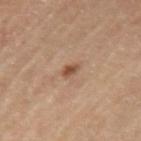{
  "lighting": "cross-polarized",
  "patient": {
    "sex": "female",
    "age_approx": 65
  },
  "lesion_size": {
    "long_diameter_mm_approx": 2.0
  },
  "image": {
    "source": "total-body photography crop",
    "field_of_view_mm": 15
  },
  "site": "arm"
}